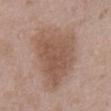follow-up: no biopsy performed (imaged during a skin exam) | imaging modality: ~15 mm crop, total-body skin-cancer survey | location: the abdomen | automated metrics: an average lesion color of about L≈54 a*≈18 b*≈27 (CIELAB), a lesion–skin lightness drop of about 9, and a normalized lesion–skin contrast near 7; border irregularity of about 3.5 on a 0–10 scale, internal color variation of about 3.5 on a 0–10 scale, and a peripheral color-asymmetry measure near 1; lesion-presence confidence of about 100/100 | diameter: ≈8 mm | tile lighting: white-light | patient: male, about 50 years old.